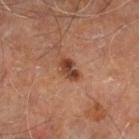{
  "biopsy_status": "not biopsied; imaged during a skin examination",
  "image": {
    "source": "total-body photography crop",
    "field_of_view_mm": 15
  },
  "lesion_size": {
    "long_diameter_mm_approx": 3.0
  },
  "automated_metrics": {
    "area_mm2_approx": 4.0,
    "eccentricity": 0.8,
    "cielab_L": 40,
    "cielab_a": 23,
    "cielab_b": 30,
    "vs_skin_darker_L": 12.0,
    "vs_skin_contrast_norm": 10.0,
    "color_variation_0_10": 4.0,
    "peripheral_color_asymmetry": 1.0
  },
  "lighting": "cross-polarized",
  "site": "right leg",
  "patient": {
    "sex": "male",
    "age_approx": 60
  }
}